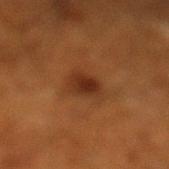<lesion>
  <biopsy_status>not biopsied; imaged during a skin examination</biopsy_status>
  <patient>
    <sex>male</sex>
    <age_approx>60</age_approx>
  </patient>
  <image>
    <source>total-body photography crop</source>
    <field_of_view_mm>15</field_of_view_mm>
  </image>
  <site>left lower leg</site>
</lesion>